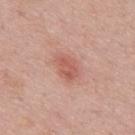Imaged during a routine full-body skin examination; the lesion was not biopsied and no histopathology is available. Cropped from a whole-body photographic skin survey; the tile spans about 15 mm. The lesion's longest dimension is about 3 mm. A male patient, in their mid- to late 40s. The lesion is located on the mid back. Captured under white-light illumination.